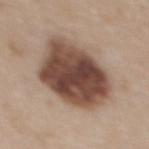Part of a total-body skin-imaging series; this lesion was reviewed on a skin check and was not flagged for biopsy. A 15 mm crop from a total-body photograph taken for skin-cancer surveillance. A female subject roughly 50 years of age. Approximately 8.5 mm at its widest. From the upper back. Imaged with white-light lighting. Automated tile analysis of the lesion measured an automated nevus-likeness rating near 75 out of 100 and lesion-presence confidence of about 100/100.